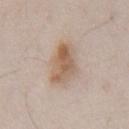{
  "biopsy_status": "not biopsied; imaged during a skin examination",
  "lesion_size": {
    "long_diameter_mm_approx": 4.5
  },
  "image": {
    "source": "total-body photography crop",
    "field_of_view_mm": 15
  },
  "lighting": "white-light",
  "patient": {
    "sex": "male",
    "age_approx": 80
  },
  "automated_metrics": {
    "cielab_L": 60,
    "cielab_a": 15,
    "cielab_b": 29,
    "vs_skin_darker_L": 10.0,
    "border_irregularity_0_10": 2.0,
    "color_variation_0_10": 6.0,
    "peripheral_color_asymmetry": 2.0,
    "nevus_likeness_0_100": 85,
    "lesion_detection_confidence_0_100": 100
  },
  "site": "abdomen"
}